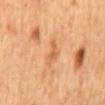Q: Was this lesion biopsied?
A: imaged on a skin check; not biopsied
Q: Automated lesion metrics?
A: a footprint of about 3.5 mm² and two-axis asymmetry of about 0.55; border irregularity of about 5 on a 0–10 scale, internal color variation of about 1 on a 0–10 scale, and a peripheral color-asymmetry measure near 0; a classifier nevus-likeness of about 0/100
Q: Lesion location?
A: the mid back
Q: How was this image acquired?
A: ~15 mm crop, total-body skin-cancer survey
Q: What is the lesion's diameter?
A: ~3 mm (longest diameter)
Q: Illumination type?
A: cross-polarized
Q: What are the patient's age and sex?
A: male, about 65 years old